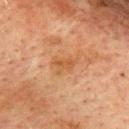Part of a total-body skin-imaging series; this lesion was reviewed on a skin check and was not flagged for biopsy.
Automated tile analysis of the lesion measured a lesion area of about 4 mm² and an eccentricity of roughly 0.75. It also reported a mean CIELAB color near L≈46 a*≈21 b*≈34 and about 6 CIELAB-L* units darker than the surrounding skin. The analysis additionally found a border-irregularity index near 4.5/10, internal color variation of about 1.5 on a 0–10 scale, and peripheral color asymmetry of about 0.5.
A region of skin cropped from a whole-body photographic capture, roughly 15 mm wide.
The lesion is located on the back.
A male patient aged 73–77.
The recorded lesion diameter is about 3 mm.
The tile uses cross-polarized illumination.